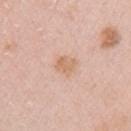Impression:
The lesion was tiled from a total-body skin photograph and was not biopsied.
Acquisition and patient details:
About 2.5 mm across. This image is a 15 mm lesion crop taken from a total-body photograph. The patient is a female approximately 40 years of age. From the arm.